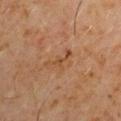tile lighting: cross-polarized illumination
patient: male, approximately 60 years of age
image source: ~15 mm tile from a whole-body skin photo
TBP lesion metrics: a lesion area of about 3.5 mm² and an outline eccentricity of about 0.9 (0 = round, 1 = elongated); a color-variation rating of about 0/10 and radial color variation of about 0; an automated nevus-likeness rating near 0 out of 100 and lesion-presence confidence of about 100/100
diameter: about 4 mm
location: the right upper arm